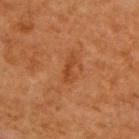Assessment: The lesion was tiled from a total-body skin photograph and was not biopsied. Clinical summary: A male subject, roughly 65 years of age. Automated image analysis of the tile measured a within-lesion color-variation index near 0/10 and a peripheral color-asymmetry measure near 0. Captured under cross-polarized illumination. A 15 mm close-up extracted from a 3D total-body photography capture. About 3.5 mm across. The lesion is on the back.